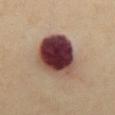{
  "biopsy_status": "not biopsied; imaged during a skin examination",
  "patient": {
    "sex": "female",
    "age_approx": 60
  },
  "image": {
    "source": "total-body photography crop",
    "field_of_view_mm": 15
  },
  "site": "front of the torso"
}